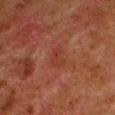site: the left lower leg | lesion size: about 3 mm | subject: male, in their 80s | image source: ~15 mm tile from a whole-body skin photo | lighting: cross-polarized illumination | automated lesion analysis: a lesion area of about 4.5 mm², an outline eccentricity of about 0.75 (0 = round, 1 = elongated), and a symmetry-axis asymmetry near 0.25; a border-irregularity index near 2.5/10 and a color-variation rating of about 2.5/10.